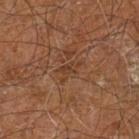workup = total-body-photography surveillance lesion; no biopsy | subject = male, aged 58–62 | imaging modality = 15 mm crop, total-body photography | body site = the right leg.